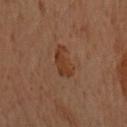The tile uses cross-polarized illumination. A female patient aged around 60. The lesion is located on the front of the torso. The recorded lesion diameter is about 3.5 mm. Automated image analysis of the tile measured a lesion area of about 7 mm², an eccentricity of roughly 0.7, and a symmetry-axis asymmetry near 0.3. The analysis additionally found an average lesion color of about L≈32 a*≈19 b*≈27 (CIELAB). The analysis additionally found a border-irregularity rating of about 3/10 and radial color variation of about 1. A roughly 15 mm field-of-view crop from a total-body skin photograph.